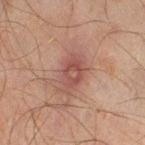Q: Was a biopsy performed?
A: imaged on a skin check; not biopsied
Q: What did automated image analysis measure?
A: a border-irregularity rating of about 2.5/10, internal color variation of about 4 on a 0–10 scale, and peripheral color asymmetry of about 1
Q: Lesion size?
A: ~4 mm (longest diameter)
Q: Lesion location?
A: the right thigh
Q: What lighting was used for the tile?
A: cross-polarized illumination
Q: What is the imaging modality?
A: 15 mm crop, total-body photography
Q: Patient demographics?
A: male, aged 43 to 47